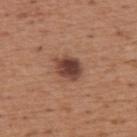Clinical impression: The lesion was photographed on a routine skin check and not biopsied; there is no pathology result. Image and clinical context: Measured at roughly 3.5 mm in maximum diameter. From the upper back. A male patient, approximately 65 years of age. A 15 mm close-up tile from a total-body photography series done for melanoma screening. Captured under white-light illumination.